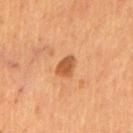{"patient": {"sex": "male", "age_approx": 55}, "automated_metrics": {"area_mm2_approx": 4.0, "eccentricity": 0.6, "shape_asymmetry": 0.3}, "lighting": "cross-polarized", "site": "chest", "lesion_size": {"long_diameter_mm_approx": 2.5}, "image": {"source": "total-body photography crop", "field_of_view_mm": 15}}